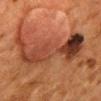Part of a total-body skin-imaging series; this lesion was reviewed on a skin check and was not flagged for biopsy. Cropped from a whole-body photographic skin survey; the tile spans about 15 mm. Captured under cross-polarized illumination. The subject is a female aged 48–52. The lesion is on the mid back. Approximately 11.5 mm at its widest.